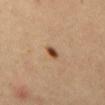The lesion was photographed on a routine skin check and not biopsied; there is no pathology result. The tile uses cross-polarized illumination. The lesion is on the abdomen. Automated tile analysis of the lesion measured an average lesion color of about L≈44 a*≈20 b*≈33 (CIELAB) and about 15 CIELAB-L* units darker than the surrounding skin. And it measured a border-irregularity index near 2/10 and a within-lesion color-variation index near 3.5/10. A male patient aged around 65. Measured at roughly 2 mm in maximum diameter. A 15 mm close-up extracted from a 3D total-body photography capture.